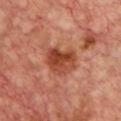Imaged during a routine full-body skin examination; the lesion was not biopsied and no histopathology is available. Captured under cross-polarized illumination. An algorithmic analysis of the crop reported a nevus-likeness score of about 5/100 and lesion-presence confidence of about 100/100. A female patient, aged 43 to 47. The lesion is on the chest. A 15 mm close-up tile from a total-body photography series done for melanoma screening. Longest diameter approximately 4 mm.